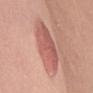Clinical impression:
No biopsy was performed on this lesion — it was imaged during a full skin examination and was not determined to be concerning.
Acquisition and patient details:
A roughly 15 mm field-of-view crop from a total-body skin photograph. Imaged with white-light lighting. A female patient about 50 years old. The lesion's longest dimension is about 6.5 mm. From the right upper arm.